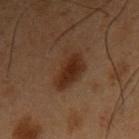<case>
<biopsy_status>not biopsied; imaged during a skin examination</biopsy_status>
<site>right upper arm</site>
<patient>
  <sex>male</sex>
  <age_approx>55</age_approx>
</patient>
<image>
  <source>total-body photography crop</source>
  <field_of_view_mm>15</field_of_view_mm>
</image>
<lighting>cross-polarized</lighting>
<lesion_size>
  <long_diameter_mm_approx>4.5</long_diameter_mm_approx>
</lesion_size>
</case>